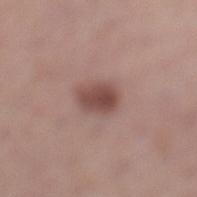About 3.5 mm across. The tile uses white-light illumination. A female patient roughly 50 years of age. A 15 mm crop from a total-body photograph taken for skin-cancer surveillance. Automated tile analysis of the lesion measured a symmetry-axis asymmetry near 0.2. It also reported a lesion color around L≈47 a*≈20 b*≈22 in CIELAB, about 12 CIELAB-L* units darker than the surrounding skin, and a normalized border contrast of about 8.5. And it measured a color-variation rating of about 3.5/10 and a peripheral color-asymmetry measure near 1.5. And it measured a lesion-detection confidence of about 100/100. The lesion is on the leg.